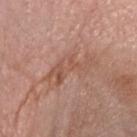This lesion was catalogued during total-body skin photography and was not selected for biopsy. The total-body-photography lesion software estimated a footprint of about 9 mm². The software also gave a border-irregularity rating of about 9.5/10, internal color variation of about 3.5 on a 0–10 scale, and radial color variation of about 1. It also reported a classifier nevus-likeness of about 0/100 and a detector confidence of about 95 out of 100 that the crop contains a lesion. This is a white-light tile. Measured at roughly 6 mm in maximum diameter. A male subject about 55 years old. A lesion tile, about 15 mm wide, cut from a 3D total-body photograph. The lesion is located on the head or neck.